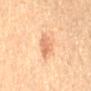Background: The lesion is located on the lower back. Automated tile analysis of the lesion measured an area of roughly 6 mm² and a symmetry-axis asymmetry near 0.25. It also reported a lesion color around L≈65 a*≈22 b*≈35 in CIELAB, a lesion–skin lightness drop of about 9, and a lesion-to-skin contrast of about 6 (normalized; higher = more distinct). The software also gave a color-variation rating of about 2.5/10 and peripheral color asymmetry of about 1. The software also gave a classifier nevus-likeness of about 30/100 and lesion-presence confidence of about 100/100. A female patient, aged 58 to 62. Imaged with cross-polarized lighting. This image is a 15 mm lesion crop taken from a total-body photograph. The recorded lesion diameter is about 3 mm.